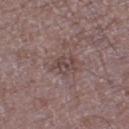follow-up — catalogued during a skin exam; not biopsied | patient — male, approximately 50 years of age | anatomic site — the left thigh | imaging modality — 15 mm crop, total-body photography | automated lesion analysis — a lesion area of about 4.5 mm², an outline eccentricity of about 0.75 (0 = round, 1 = elongated), and a symmetry-axis asymmetry near 0.35; a classifier nevus-likeness of about 0/100 and lesion-presence confidence of about 65/100 | size — ≈3 mm | illumination — white-light.